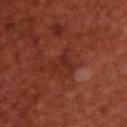The tile uses cross-polarized illumination. A male subject aged around 50. A roughly 15 mm field-of-view crop from a total-body skin photograph. Approximately 3 mm at its widest. Located on the upper back.